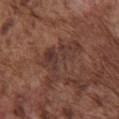Impression:
Captured during whole-body skin photography for melanoma surveillance; the lesion was not biopsied.
Background:
The tile uses white-light illumination. The lesion is located on the chest. Longest diameter approximately 6.5 mm. Cropped from a total-body skin-imaging series; the visible field is about 15 mm. A male subject in their mid-70s.